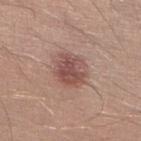Assessment: Part of a total-body skin-imaging series; this lesion was reviewed on a skin check and was not flagged for biopsy. Image and clinical context: The lesion is located on the right lower leg. This is a white-light tile. A 15 mm close-up extracted from a 3D total-body photography capture. Measured at roughly 4 mm in maximum diameter. The subject is a male aged approximately 30. Automated image analysis of the tile measured an average lesion color of about L≈50 a*≈21 b*≈23 (CIELAB), roughly 11 lightness units darker than nearby skin, and a lesion-to-skin contrast of about 7.5 (normalized; higher = more distinct).